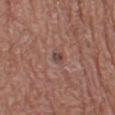This lesion was catalogued during total-body skin photography and was not selected for biopsy. An algorithmic analysis of the crop reported an automated nevus-likeness rating near 0 out of 100 and a lesion-detection confidence of about 100/100. A female subject aged 48–52. This is a white-light tile. A close-up tile cropped from a whole-body skin photograph, about 15 mm across. Located on the left thigh. About 2 mm across.